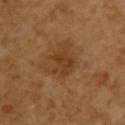| field | value |
|---|---|
| biopsy status | imaged on a skin check; not biopsied |
| imaging modality | ~15 mm tile from a whole-body skin photo |
| subject | female, aged 53–57 |
| body site | the upper back |
| automated lesion analysis | a lesion–skin lightness drop of about 7 and a lesion-to-skin contrast of about 6.5 (normalized; higher = more distinct); a border-irregularity index near 5/10, internal color variation of about 2.5 on a 0–10 scale, and peripheral color asymmetry of about 1 |
| lesion diameter | about 4.5 mm |
| lighting | cross-polarized illumination |